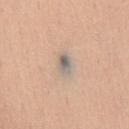Notes:
- workup: imaged on a skin check; not biopsied
- acquisition: total-body-photography crop, ~15 mm field of view
- anatomic site: the chest
- subject: female, in their mid-50s